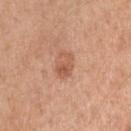  site: right upper arm
  lesion_size:
    long_diameter_mm_approx: 3.0
  lighting: white-light
  patient:
    sex: female
    age_approx: 40
  image:
    source: total-body photography crop
    field_of_view_mm: 15